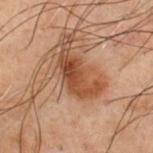This lesion was catalogued during total-body skin photography and was not selected for biopsy. A 15 mm crop from a total-body photograph taken for skin-cancer surveillance. Measured at roughly 5.5 mm in maximum diameter. Captured under cross-polarized illumination. An algorithmic analysis of the crop reported an average lesion color of about L≈35 a*≈19 b*≈27 (CIELAB) and about 10 CIELAB-L* units darker than the surrounding skin. And it measured a color-variation rating of about 5/10 and radial color variation of about 2. On the front of the torso. A male patient aged around 70.